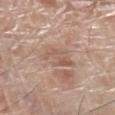– workup: catalogued during a skin exam; not biopsied